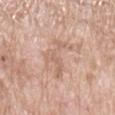biopsy_status: not biopsied; imaged during a skin examination
lesion_size:
  long_diameter_mm_approx: 6.0
site: left upper arm
patient:
  sex: female
  age_approx: 70
image:
  source: total-body photography crop
  field_of_view_mm: 15
lighting: white-light